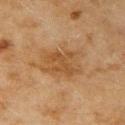Captured during whole-body skin photography for melanoma surveillance; the lesion was not biopsied. A roughly 15 mm field-of-view crop from a total-body skin photograph. Approximately 5.5 mm at its widest. Imaged with cross-polarized lighting. A female patient, aged 58 to 62. The lesion is located on the right upper arm.